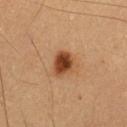Clinical impression:
Part of a total-body skin-imaging series; this lesion was reviewed on a skin check and was not flagged for biopsy.
Image and clinical context:
An algorithmic analysis of the crop reported an outline eccentricity of about 0.45 (0 = round, 1 = elongated) and a symmetry-axis asymmetry near 0.2. It also reported a mean CIELAB color near L≈39 a*≈22 b*≈32, about 15 CIELAB-L* units darker than the surrounding skin, and a lesion-to-skin contrast of about 11.5 (normalized; higher = more distinct). The software also gave a border-irregularity rating of about 2/10, internal color variation of about 4.5 on a 0–10 scale, and a peripheral color-asymmetry measure near 1.5. The analysis additionally found a detector confidence of about 100 out of 100 that the crop contains a lesion. On the lower back. This is a cross-polarized tile. A male subject, in their 60s. Measured at roughly 3 mm in maximum diameter. This image is a 15 mm lesion crop taken from a total-body photograph.